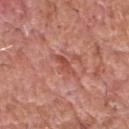Recorded during total-body skin imaging; not selected for excision or biopsy. Cropped from a total-body skin-imaging series; the visible field is about 15 mm. Longest diameter approximately 3 mm. From the head or neck. Captured under white-light illumination. A male patient, roughly 60 years of age.